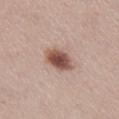{
  "biopsy_status": "not biopsied; imaged during a skin examination",
  "image": {
    "source": "total-body photography crop",
    "field_of_view_mm": 15
  },
  "automated_metrics": {
    "eccentricity": 0.75,
    "shape_asymmetry": 0.15,
    "vs_skin_darker_L": 16.0,
    "border_irregularity_0_10": 1.5,
    "color_variation_0_10": 5.0,
    "peripheral_color_asymmetry": 1.0
  },
  "lesion_size": {
    "long_diameter_mm_approx": 4.0
  },
  "patient": {
    "sex": "male",
    "age_approx": 60
  },
  "site": "leg"
}